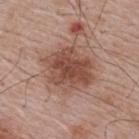Case summary:
• anatomic site · the upper back
• patient · male, approximately 65 years of age
• acquisition · ~15 mm crop, total-body skin-cancer survey
• automated metrics · a mean CIELAB color near L≈48 a*≈21 b*≈27 and a normalized lesion–skin contrast near 8.5; a border-irregularity index near 2.5/10, a within-lesion color-variation index near 4.5/10, and peripheral color asymmetry of about 1.5
• diameter · ~5.5 mm (longest diameter)